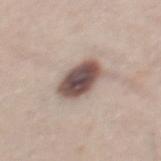– anatomic site — the mid back
– acquisition — ~15 mm tile from a whole-body skin photo
– patient — male, aged 33–37
– illumination — white-light